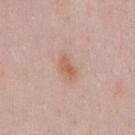{"biopsy_status": "not biopsied; imaged during a skin examination", "image": {"source": "total-body photography crop", "field_of_view_mm": 15}, "site": "chest", "patient": {"sex": "male", "age_approx": 55}}